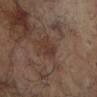Recorded during total-body skin imaging; not selected for excision or biopsy. From the arm. Automated tile analysis of the lesion measured an area of roughly 4.5 mm², a shape eccentricity near 0.6, and a symmetry-axis asymmetry near 0.25. And it measured an average lesion color of about L≈31 a*≈16 b*≈21 (CIELAB), a lesion–skin lightness drop of about 5, and a lesion-to-skin contrast of about 5.5 (normalized; higher = more distinct). A female patient, roughly 80 years of age. Longest diameter approximately 2.5 mm. A roughly 15 mm field-of-view crop from a total-body skin photograph.